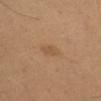No biopsy was performed on this lesion — it was imaged during a full skin examination and was not determined to be concerning.
The lesion is located on the left upper arm.
A 15 mm crop from a total-body photograph taken for skin-cancer surveillance.
Approximately 3 mm at its widest.
A male subject aged 48 to 52.